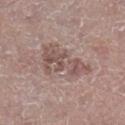Context: Automated image analysis of the tile measured border irregularity of about 7.5 on a 0–10 scale, a color-variation rating of about 4.5/10, and radial color variation of about 1.5. It also reported a nevus-likeness score of about 0/100 and a detector confidence of about 100 out of 100 that the crop contains a lesion. On the leg. Measured at roughly 6.5 mm in maximum diameter. Cropped from a total-body skin-imaging series; the visible field is about 15 mm. This is a white-light tile. A male patient, about 65 years old.